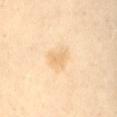Assessment: Recorded during total-body skin imaging; not selected for excision or biopsy. Context: A region of skin cropped from a whole-body photographic capture, roughly 15 mm wide. The subject is a female in their mid-50s. The lesion is located on the abdomen. Measured at roughly 2.5 mm in maximum diameter.